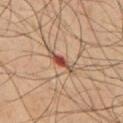follow-up: total-body-photography surveillance lesion; no biopsy.